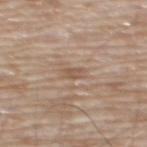| field | value |
|---|---|
| follow-up | no biopsy performed (imaged during a skin exam) |
| acquisition | ~15 mm tile from a whole-body skin photo |
| anatomic site | the mid back |
| automated lesion analysis | an area of roughly 3 mm², a shape eccentricity near 0.85, and a symmetry-axis asymmetry near 0.3; an average lesion color of about L≈54 a*≈16 b*≈28 (CIELAB), a lesion–skin lightness drop of about 7, and a normalized lesion–skin contrast near 5.5; a classifier nevus-likeness of about 0/100 and a lesion-detection confidence of about 95/100 |
| subject | male, in their 80s |
| lesion diameter | ≈2.5 mm |